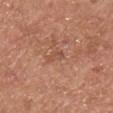Notes:
– follow-up — catalogued during a skin exam; not biopsied
– lesion size — ~2.5 mm (longest diameter)
– image source — 15 mm crop, total-body photography
– illumination — white-light illumination
– anatomic site — the chest
– patient — male, approximately 75 years of age
– TBP lesion metrics — an eccentricity of roughly 0.9 and a shape-asymmetry score of about 0.45 (0 = symmetric)